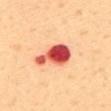biopsy status=no biopsy performed (imaged during a skin exam)
image=15 mm crop, total-body photography
location=the back
subject=female, aged around 50
illumination=cross-polarized illumination